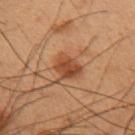{
  "site": "arm",
  "patient": {
    "sex": "male",
    "age_approx": 55
  },
  "lighting": "cross-polarized",
  "image": {
    "source": "total-body photography crop",
    "field_of_view_mm": 15
  },
  "automated_metrics": {
    "vs_skin_darker_L": 9.0,
    "vs_skin_contrast_norm": 8.0
  }
}